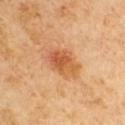Q: Is there a histopathology result?
A: imaged on a skin check; not biopsied
Q: What lighting was used for the tile?
A: cross-polarized
Q: How was this image acquired?
A: ~15 mm tile from a whole-body skin photo
Q: Patient demographics?
A: male, aged around 65
Q: What is the lesion's diameter?
A: ≈4.5 mm
Q: Automated lesion metrics?
A: an eccentricity of roughly 0.75 and a shape-asymmetry score of about 0.2 (0 = symmetric); a lesion color around L≈56 a*≈26 b*≈40 in CIELAB, about 11 CIELAB-L* units darker than the surrounding skin, and a normalized border contrast of about 7.5; border irregularity of about 2.5 on a 0–10 scale and peripheral color asymmetry of about 2.5; a nevus-likeness score of about 90/100 and a lesion-detection confidence of about 100/100